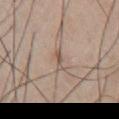Findings:
– workup · imaged on a skin check; not biopsied
– lighting · white-light
– image · 15 mm crop, total-body photography
– size · ≈2.5 mm
– site · the chest
– patient · male, about 75 years old